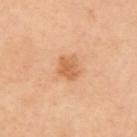Case summary:
- biopsy status — imaged on a skin check; not biopsied
- size — ≈3 mm
- image source — 15 mm crop, total-body photography
- body site — the left upper arm
- TBP lesion metrics — an area of roughly 5 mm² and a symmetry-axis asymmetry near 0.35; a lesion color around L≈62 a*≈25 b*≈40 in CIELAB
- lighting — cross-polarized illumination
- patient — male, in their mid- to late 60s